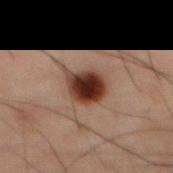The lesion was photographed on a routine skin check and not biopsied; there is no pathology result.
Cropped from a total-body skin-imaging series; the visible field is about 15 mm.
Measured at roughly 4 mm in maximum diameter.
A male subject roughly 60 years of age.
The lesion is located on the front of the torso.